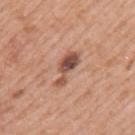patient=female, in their 40s; image source=total-body-photography crop, ~15 mm field of view; location=the left upper arm; illumination=white-light.